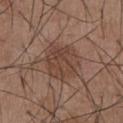Recorded during total-body skin imaging; not selected for excision or biopsy.
Cropped from a whole-body photographic skin survey; the tile spans about 15 mm.
A male patient, about 55 years old.
On the abdomen.
Automated image analysis of the tile measured an eccentricity of roughly 0.55. The software also gave a lesion–skin lightness drop of about 8 and a normalized lesion–skin contrast near 6.5. The analysis additionally found an automated nevus-likeness rating near 15 out of 100.
This is a white-light tile.
Approximately 5 mm at its widest.